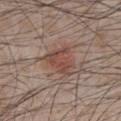{
  "biopsy_status": "not biopsied; imaged during a skin examination",
  "patient": {
    "sex": "male",
    "age_approx": 65
  },
  "image": {
    "source": "total-body photography crop",
    "field_of_view_mm": 15
  },
  "site": "chest"
}